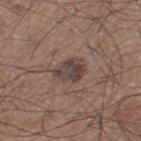Impression: The lesion was tiled from a total-body skin photograph and was not biopsied. Image and clinical context: The tile uses white-light illumination. A male patient, aged approximately 50. The lesion-visualizer software estimated a lesion area of about 7.5 mm² and a symmetry-axis asymmetry near 0.25. The analysis additionally found an average lesion color of about L≈41 a*≈15 b*≈18 (CIELAB), roughly 10 lightness units darker than nearby skin, and a normalized border contrast of about 8.5. And it measured a border-irregularity rating of about 2.5/10, a color-variation rating of about 5/10, and radial color variation of about 1.5. A roughly 15 mm field-of-view crop from a total-body skin photograph. Located on the left thigh. Longest diameter approximately 3.5 mm.